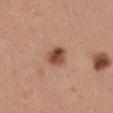<lesion>
  <biopsy_status>not biopsied; imaged during a skin examination</biopsy_status>
  <image>
    <source>total-body photography crop</source>
    <field_of_view_mm>15</field_of_view_mm>
  </image>
  <lesion_size>
    <long_diameter_mm_approx>3.0</long_diameter_mm_approx>
  </lesion_size>
  <patient>
    <sex>female</sex>
    <age_approx>30</age_approx>
  </patient>
  <site>leg</site>
</lesion>